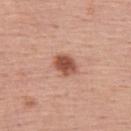The lesion was photographed on a routine skin check and not biopsied; there is no pathology result.
A male patient approximately 55 years of age.
A lesion tile, about 15 mm wide, cut from a 3D total-body photograph.
From the upper back.
This is a white-light tile.
Longest diameter approximately 3 mm.